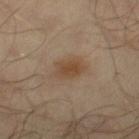workup = total-body-photography surveillance lesion; no biopsy | image-analysis metrics = an eccentricity of roughly 0.75 and a symmetry-axis asymmetry near 0.2; a border-irregularity index near 2/10 and a within-lesion color-variation index near 2.5/10 | acquisition = ~15 mm tile from a whole-body skin photo | site = the leg | lighting = cross-polarized | subject = male, approximately 65 years of age | lesion diameter = about 3.5 mm.